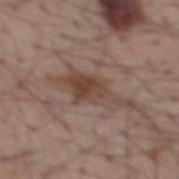No biopsy was performed on this lesion — it was imaged during a full skin examination and was not determined to be concerning. The lesion is on the mid back. The subject is a male aged 58 to 62. A roughly 15 mm field-of-view crop from a total-body skin photograph. Captured under white-light illumination.